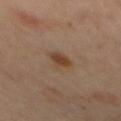The lesion was photographed on a routine skin check and not biopsied; there is no pathology result. A roughly 15 mm field-of-view crop from a total-body skin photograph. This is a cross-polarized tile. On the mid back. The patient is a female aged 63 to 67.